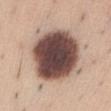<record>
  <biopsy_status>not biopsied; imaged during a skin examination</biopsy_status>
  <image>
    <source>total-body photography crop</source>
    <field_of_view_mm>15</field_of_view_mm>
  </image>
  <lesion_size>
    <long_diameter_mm_approx>8.0</long_diameter_mm_approx>
  </lesion_size>
  <site>front of the torso</site>
  <automated_metrics>
    <cielab_L>45</cielab_L>
    <cielab_a>18</cielab_a>
    <cielab_b>21</cielab_b>
    <vs_skin_darker_L>25.0</vs_skin_darker_L>
    <vs_skin_contrast_norm>16.5</vs_skin_contrast_norm>
    <nevus_likeness_0_100>35</nevus_likeness_0_100>
    <lesion_detection_confidence_0_100>100</lesion_detection_confidence_0_100>
  </automated_metrics>
  <patient>
    <sex>male</sex>
    <age_approx>35</age_approx>
  </patient>
</record>